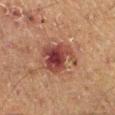Recorded during total-body skin imaging; not selected for excision or biopsy.